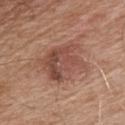The lesion was photographed on a routine skin check and not biopsied; there is no pathology result. This image is a 15 mm lesion crop taken from a total-body photograph. From the chest. The subject is a male aged approximately 55. Measured at roughly 6 mm in maximum diameter. Captured under white-light illumination.